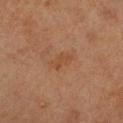The lesion was tiled from a total-body skin photograph and was not biopsied. A 15 mm close-up extracted from a 3D total-body photography capture. The recorded lesion diameter is about 3 mm. Located on the chest. Captured under cross-polarized illumination. A female subject, aged around 45.